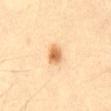<case>
  <biopsy_status>not biopsied; imaged during a skin examination</biopsy_status>
  <automated_metrics>
    <shape_asymmetry>0.25</shape_asymmetry>
    <lesion_detection_confidence_0_100>100</lesion_detection_confidence_0_100>
  </automated_metrics>
  <image>
    <source>total-body photography crop</source>
    <field_of_view_mm>15</field_of_view_mm>
  </image>
  <site>abdomen</site>
  <patient>
    <sex>male</sex>
    <age_approx>35</age_approx>
  </patient>
</case>